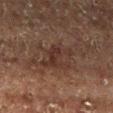The lesion was photographed on a routine skin check and not biopsied; there is no pathology result. The patient is a male in their mid-70s. A 15 mm crop from a total-body photograph taken for skin-cancer surveillance. The lesion is located on the left lower leg.